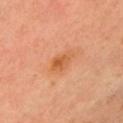Part of a total-body skin-imaging series; this lesion was reviewed on a skin check and was not flagged for biopsy.
Imaged with cross-polarized lighting.
The total-body-photography lesion software estimated an area of roughly 5.5 mm² and a shape eccentricity near 0.85. And it measured an automated nevus-likeness rating near 15 out of 100 and a detector confidence of about 100 out of 100 that the crop contains a lesion.
Located on the chest.
A female patient approximately 40 years of age.
A roughly 15 mm field-of-view crop from a total-body skin photograph.